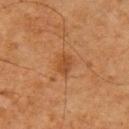Impression:
Recorded during total-body skin imaging; not selected for excision or biopsy.
Background:
The patient is a male aged 58–62. An algorithmic analysis of the crop reported a classifier nevus-likeness of about 45/100. A close-up tile cropped from a whole-body skin photograph, about 15 mm across. The lesion is on the left upper arm. Measured at roughly 2.5 mm in maximum diameter. Captured under cross-polarized illumination.